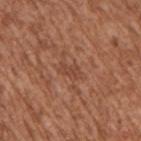| field | value |
|---|---|
| location | the right upper arm |
| size | about 2.5 mm |
| illumination | white-light illumination |
| imaging modality | ~15 mm crop, total-body skin-cancer survey |
| subject | male, aged 43 to 47 |